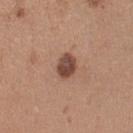This lesion was catalogued during total-body skin photography and was not selected for biopsy. On the right upper arm. Measured at roughly 3.5 mm in maximum diameter. A 15 mm close-up tile from a total-body photography series done for melanoma screening. Captured under white-light illumination. A female patient approximately 30 years of age. Automated image analysis of the tile measured an eccentricity of roughly 0.7. The analysis additionally found a lesion-detection confidence of about 100/100.